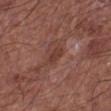Captured during whole-body skin photography for melanoma surveillance; the lesion was not biopsied. A 15 mm crop from a total-body photograph taken for skin-cancer surveillance. The lesion is located on the left lower leg. A male patient, in their mid- to late 60s.